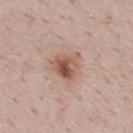workup = imaged on a skin check; not biopsied
lesion size = about 3.5 mm
patient = male, approximately 50 years of age
image source = ~15 mm tile from a whole-body skin photo
location = the back